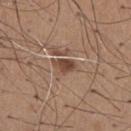{"patient": {"sex": "male", "age_approx": 65}, "site": "chest", "lighting": "white-light", "image": {"source": "total-body photography crop", "field_of_view_mm": 15}}